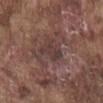Background:
Cropped from a total-body skin-imaging series; the visible field is about 15 mm. The lesion is on the chest. This is a white-light tile. The patient is a male aged approximately 75.